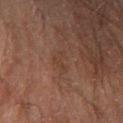Assessment: Recorded during total-body skin imaging; not selected for excision or biopsy. Context: A male patient, approximately 65 years of age. A region of skin cropped from a whole-body photographic capture, roughly 15 mm wide. The lesion is located on the right forearm.